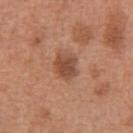This lesion was catalogued during total-body skin photography and was not selected for biopsy.
The total-body-photography lesion software estimated a footprint of about 6.5 mm², an outline eccentricity of about 0.35 (0 = round, 1 = elongated), and a symmetry-axis asymmetry near 0.25. The software also gave border irregularity of about 2 on a 0–10 scale and a peripheral color-asymmetry measure near 1. And it measured an automated nevus-likeness rating near 40 out of 100 and a lesion-detection confidence of about 100/100.
A male patient, about 65 years old.
The recorded lesion diameter is about 3 mm.
The lesion is located on the abdomen.
A 15 mm close-up extracted from a 3D total-body photography capture.
The tile uses white-light illumination.